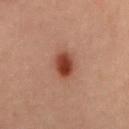<record>
<biopsy_status>not biopsied; imaged during a skin examination</biopsy_status>
<image>
  <source>total-body photography crop</source>
  <field_of_view_mm>15</field_of_view_mm>
</image>
<site>mid back</site>
<patient>
  <sex>male</sex>
  <age_approx>40</age_approx>
</patient>
</record>